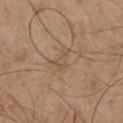Captured during whole-body skin photography for melanoma surveillance; the lesion was not biopsied.